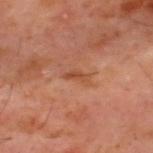| field | value |
|---|---|
| patient | male, in their 60s |
| imaging modality | ~15 mm crop, total-body skin-cancer survey |
| illumination | cross-polarized |
| location | the upper back |
| diameter | ~2.5 mm (longest diameter) |